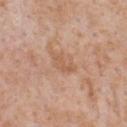No biopsy was performed on this lesion — it was imaged during a full skin examination and was not determined to be concerning.
A 15 mm close-up extracted from a 3D total-body photography capture.
A male patient, approximately 65 years of age.
Located on the front of the torso.